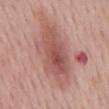Clinical impression: Recorded during total-body skin imaging; not selected for excision or biopsy. Acquisition and patient details: About 8.5 mm across. A 15 mm crop from a total-body photograph taken for skin-cancer surveillance. The patient is a female aged around 65. The lesion is located on the mid back.